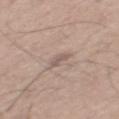Assessment: Part of a total-body skin-imaging series; this lesion was reviewed on a skin check and was not flagged for biopsy. Acquisition and patient details: The lesion is on the mid back. Imaged with white-light lighting. A 15 mm close-up tile from a total-body photography series done for melanoma screening. A male subject, aged 63–67.